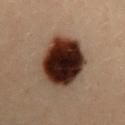Conclusion: Histopathological examination showed a dysplastic (Clark) nevus — a benign lesion.